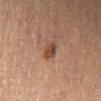Recorded during total-body skin imaging; not selected for excision or biopsy.
About 2.5 mm across.
This is a cross-polarized tile.
Automated image analysis of the tile measured an average lesion color of about L≈39 a*≈19 b*≈27 (CIELAB) and roughly 9 lightness units darker than nearby skin.
Cropped from a total-body skin-imaging series; the visible field is about 15 mm.
From the leg.
A male subject aged 83 or over.